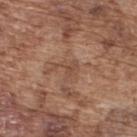Captured during whole-body skin photography for melanoma surveillance; the lesion was not biopsied. The total-body-photography lesion software estimated a symmetry-axis asymmetry near 0.65. It also reported a mean CIELAB color near L≈48 a*≈19 b*≈29, roughly 7 lightness units darker than nearby skin, and a normalized border contrast of about 5.5. The analysis additionally found internal color variation of about 1 on a 0–10 scale. A close-up tile cropped from a whole-body skin photograph, about 15 mm across. On the upper back. Captured under white-light illumination. A male subject in their mid- to late 70s. Approximately 3 mm at its widest.